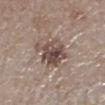Imaged during a routine full-body skin examination; the lesion was not biopsied and no histopathology is available. A lesion tile, about 15 mm wide, cut from a 3D total-body photograph. Captured under white-light illumination. A female patient in their mid-50s. The recorded lesion diameter is about 4 mm. An algorithmic analysis of the crop reported a lesion area of about 12 mm², an outline eccentricity of about 0.45 (0 = round, 1 = elongated), and a shape-asymmetry score of about 0.3 (0 = symmetric). And it measured an average lesion color of about L≈47 a*≈15 b*≈21 (CIELAB), a lesion–skin lightness drop of about 12, and a normalized border contrast of about 9. The software also gave border irregularity of about 4 on a 0–10 scale, a color-variation rating of about 7.5/10, and peripheral color asymmetry of about 3. The lesion is located on the left lower leg.